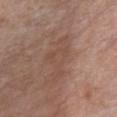The lesion was tiled from a total-body skin photograph and was not biopsied. Located on the chest. The recorded lesion diameter is about 6 mm. A female patient, in their mid-60s. A lesion tile, about 15 mm wide, cut from a 3D total-body photograph.